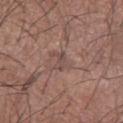The lesion was tiled from a total-body skin photograph and was not biopsied. The subject is a male roughly 65 years of age. This image is a 15 mm lesion crop taken from a total-body photograph. Automated tile analysis of the lesion measured a lesion color around L≈47 a*≈17 b*≈21 in CIELAB and a lesion–skin lightness drop of about 7. The analysis additionally found a border-irregularity rating of about 3.5/10, a color-variation rating of about 0/10, and peripheral color asymmetry of about 0. The analysis additionally found a nevus-likeness score of about 0/100 and a detector confidence of about 80 out of 100 that the crop contains a lesion. This is a white-light tile. The lesion is on the left forearm. Measured at roughly 2.5 mm in maximum diameter.